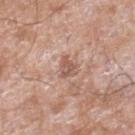workup: imaged on a skin check; not biopsied
TBP lesion metrics: an outline eccentricity of about 0.65 (0 = round, 1 = elongated) and a shape-asymmetry score of about 0.4 (0 = symmetric); a border-irregularity rating of about 4/10 and radial color variation of about 1
acquisition: ~15 mm tile from a whole-body skin photo
location: the leg
tile lighting: white-light illumination
subject: male, in their 60s